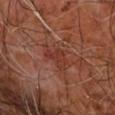No biopsy was performed on this lesion — it was imaged during a full skin examination and was not determined to be concerning. A patient roughly 65 years of age. The lesion is located on the right forearm. A 15 mm crop from a total-body photograph taken for skin-cancer surveillance.